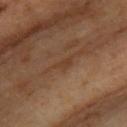{
  "biopsy_status": "not biopsied; imaged during a skin examination",
  "lighting": "cross-polarized",
  "site": "upper back",
  "patient": {
    "sex": "male",
    "age_approx": 60
  },
  "image": {
    "source": "total-body photography crop",
    "field_of_view_mm": 15
  }
}